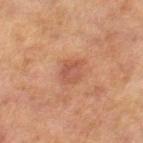| key | value |
|---|---|
| workup | no biopsy performed (imaged during a skin exam) |
| TBP lesion metrics | an outline eccentricity of about 0.55 (0 = round, 1 = elongated); a lesion color around L≈47 a*≈23 b*≈29 in CIELAB, roughly 7 lightness units darker than nearby skin, and a normalized lesion–skin contrast near 5.5; a border-irregularity index near 2/10, a within-lesion color-variation index near 3/10, and peripheral color asymmetry of about 1; a nevus-likeness score of about 30/100 |
| body site | the right thigh |
| diameter | ≈3 mm |
| imaging modality | ~15 mm tile from a whole-body skin photo |
| tile lighting | cross-polarized |
| subject | female, roughly 60 years of age |